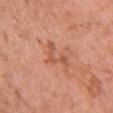Background:
This image is a 15 mm lesion crop taken from a total-body photograph. A female subject approximately 70 years of age. From the upper back.